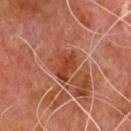{
  "biopsy_status": "not biopsied; imaged during a skin examination",
  "patient": {
    "sex": "male",
    "age_approx": 65
  },
  "lesion_size": {
    "long_diameter_mm_approx": 3.5
  },
  "image": {
    "source": "total-body photography crop",
    "field_of_view_mm": 15
  },
  "site": "chest",
  "automated_metrics": {
    "area_mm2_approx": 6.5,
    "eccentricity": 0.75,
    "shape_asymmetry": 0.3,
    "cielab_L": 44,
    "cielab_a": 30,
    "cielab_b": 36,
    "vs_skin_contrast_norm": 8.0,
    "border_irregularity_0_10": 3.5,
    "color_variation_0_10": 2.5,
    "peripheral_color_asymmetry": 1.0
  }
}